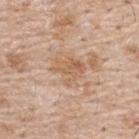This lesion was catalogued during total-body skin photography and was not selected for biopsy. Imaged with white-light lighting. The lesion is on the upper back. The subject is a male about 55 years old. An algorithmic analysis of the crop reported a lesion color around L≈60 a*≈19 b*≈33 in CIELAB and roughly 8 lightness units darker than nearby skin. The analysis additionally found a classifier nevus-likeness of about 0/100 and lesion-presence confidence of about 95/100. A close-up tile cropped from a whole-body skin photograph, about 15 mm across.